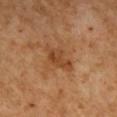{
  "biopsy_status": "not biopsied; imaged during a skin examination",
  "lighting": "cross-polarized",
  "patient": {
    "sex": "female",
    "age_approx": 50
  },
  "image": {
    "source": "total-body photography crop",
    "field_of_view_mm": 15
  },
  "lesion_size": {
    "long_diameter_mm_approx": 4.0
  },
  "site": "right lower leg",
  "automated_metrics": {
    "area_mm2_approx": 7.0,
    "eccentricity": 0.8,
    "shape_asymmetry": 0.3,
    "cielab_L": 46,
    "cielab_a": 24,
    "cielab_b": 38,
    "vs_skin_darker_L": 8.0,
    "vs_skin_contrast_norm": 6.5,
    "color_variation_0_10": 3.0,
    "peripheral_color_asymmetry": 1.0
  }
}